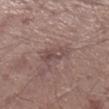The lesion was tiled from a total-body skin photograph and was not biopsied. The patient is a male about 60 years old. Located on the right lower leg. Approximately 3.5 mm at its widest. Cropped from a total-body skin-imaging series; the visible field is about 15 mm. Captured under white-light illumination.